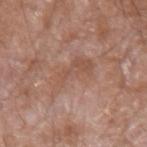Q: Was this lesion biopsied?
A: catalogued during a skin exam; not biopsied
Q: Where on the body is the lesion?
A: the left forearm
Q: Automated lesion metrics?
A: an eccentricity of roughly 0.9 and two-axis asymmetry of about 0.45; a lesion color around L≈52 a*≈20 b*≈28 in CIELAB, about 7 CIELAB-L* units darker than the surrounding skin, and a normalized lesion–skin contrast near 5; an automated nevus-likeness rating near 0 out of 100 and a detector confidence of about 100 out of 100 that the crop contains a lesion
Q: What is the imaging modality?
A: ~15 mm tile from a whole-body skin photo
Q: How large is the lesion?
A: ≈5.5 mm
Q: Who is the patient?
A: male, approximately 60 years of age